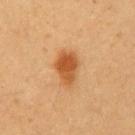| key | value |
|---|---|
| biopsy status | no biopsy performed (imaged during a skin exam) |
| body site | the chest |
| automated lesion analysis | a footprint of about 8.5 mm², an eccentricity of roughly 0.8, and a shape-asymmetry score of about 0.2 (0 = symmetric); a lesion color around L≈51 a*≈25 b*≈41 in CIELAB and a lesion–skin lightness drop of about 12; a border-irregularity index near 2/10, a color-variation rating of about 4/10, and a peripheral color-asymmetry measure near 1.5 |
| tile lighting | cross-polarized |
| patient | male, roughly 45 years of age |
| image | 15 mm crop, total-body photography |